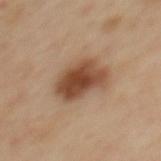notes = no biopsy performed (imaged during a skin exam) | tile lighting = cross-polarized | location = the back | imaging modality = total-body-photography crop, ~15 mm field of view | image-analysis metrics = a mean CIELAB color near L≈48 a*≈21 b*≈32, roughly 15 lightness units darker than nearby skin, and a normalized border contrast of about 10.5; a border-irregularity rating of about 2.5/10, a within-lesion color-variation index near 5/10, and a peripheral color-asymmetry measure near 1.5 | subject = female, aged 38–42 | diameter = about 5.5 mm.